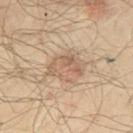follow-up: total-body-photography surveillance lesion; no biopsy | lesion size: about 4.5 mm | tile lighting: cross-polarized illumination | body site: the chest | acquisition: total-body-photography crop, ~15 mm field of view | patient: male, aged around 50.